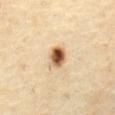Assessment:
No biopsy was performed on this lesion — it was imaged during a full skin examination and was not determined to be concerning.
Clinical summary:
This image is a 15 mm lesion crop taken from a total-body photograph. The total-body-photography lesion software estimated a lesion color around L≈56 a*≈18 b*≈35 in CIELAB, about 19 CIELAB-L* units darker than the surrounding skin, and a normalized lesion–skin contrast near 12. It also reported a nevus-likeness score of about 100/100 and lesion-presence confidence of about 100/100. Captured under cross-polarized illumination. The recorded lesion diameter is about 3 mm. A male subject, aged 68 to 72. From the abdomen.